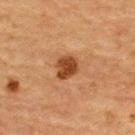Case summary:
• biopsy status: imaged on a skin check; not biopsied
• illumination: cross-polarized illumination
• size: ≈3 mm
• image source: ~15 mm crop, total-body skin-cancer survey
• site: the upper back
• image-analysis metrics: border irregularity of about 2 on a 0–10 scale, a within-lesion color-variation index near 2.5/10, and radial color variation of about 1
• patient: female, aged approximately 70